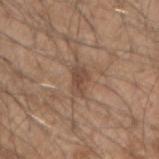biopsy status: imaged on a skin check; not biopsied
acquisition: 15 mm crop, total-body photography
patient: male, approximately 50 years of age
location: the arm
illumination: white-light illumination